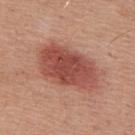Q: Was a biopsy performed?
A: no biopsy performed (imaged during a skin exam)